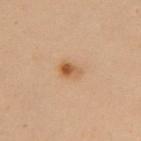This lesion was catalogued during total-body skin photography and was not selected for biopsy.
A female subject, aged around 50.
This is a cross-polarized tile.
The lesion is on the chest.
The lesion-visualizer software estimated an average lesion color of about L≈49 a*≈18 b*≈33 (CIELAB) and a normalized border contrast of about 7.5. The analysis additionally found border irregularity of about 2 on a 0–10 scale, a color-variation rating of about 7.5/10, and peripheral color asymmetry of about 2.5. And it measured an automated nevus-likeness rating near 95 out of 100 and lesion-presence confidence of about 100/100.
A close-up tile cropped from a whole-body skin photograph, about 15 mm across.
Longest diameter approximately 3 mm.